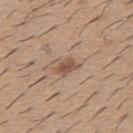| key | value |
|---|---|
| notes | no biopsy performed (imaged during a skin exam) |
| lesion size | ~3 mm (longest diameter) |
| patient | male, aged approximately 60 |
| location | the upper back |
| image | ~15 mm tile from a whole-body skin photo |
| lighting | white-light |